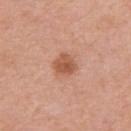Captured during whole-body skin photography for melanoma surveillance; the lesion was not biopsied.
The lesion's longest dimension is about 2.5 mm.
The subject is a female aged approximately 40.
A 15 mm close-up extracted from a 3D total-body photography capture.
On the right upper arm.
Imaged with white-light lighting.